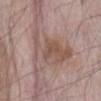Impression:
The lesion was photographed on a routine skin check and not biopsied; there is no pathology result.
Clinical summary:
A male subject, aged around 70. Located on the abdomen. A close-up tile cropped from a whole-body skin photograph, about 15 mm across.